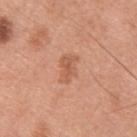biopsy status — total-body-photography surveillance lesion; no biopsy
image-analysis metrics — a footprint of about 6 mm², an outline eccentricity of about 0.8 (0 = round, 1 = elongated), and two-axis asymmetry of about 0.35; a border-irregularity rating of about 3.5/10, a color-variation rating of about 3.5/10, and a peripheral color-asymmetry measure near 1
imaging modality — ~15 mm crop, total-body skin-cancer survey
size — about 3.5 mm
site — the back
tile lighting — white-light illumination
patient — male, approximately 30 years of age